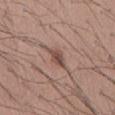  biopsy_status: not biopsied; imaged during a skin examination
  site: mid back
  patient:
    sex: male
    age_approx: 40
  image:
    source: total-body photography crop
    field_of_view_mm: 15